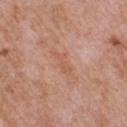This is a white-light tile. Longest diameter approximately 3.5 mm. A 15 mm close-up tile from a total-body photography series done for melanoma screening. On the chest. The patient is a male roughly 60 years of age.